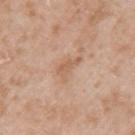follow-up = catalogued during a skin exam; not biopsied
image = 15 mm crop, total-body photography
site = the left upper arm
illumination = white-light illumination
diameter = about 3.5 mm
patient = male, in their 50s